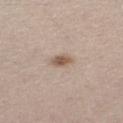Impression: Part of a total-body skin-imaging series; this lesion was reviewed on a skin check and was not flagged for biopsy. Context: The lesion's longest dimension is about 2.5 mm. A female patient aged around 40. A 15 mm crop from a total-body photograph taken for skin-cancer surveillance. An algorithmic analysis of the crop reported a lesion color around L≈56 a*≈15 b*≈26 in CIELAB, about 11 CIELAB-L* units darker than the surrounding skin, and a normalized lesion–skin contrast near 8. It also reported a border-irregularity index near 2.5/10, internal color variation of about 5 on a 0–10 scale, and a peripheral color-asymmetry measure near 2. The analysis additionally found an automated nevus-likeness rating near 90 out of 100 and a detector confidence of about 100 out of 100 that the crop contains a lesion. The lesion is located on the left thigh.